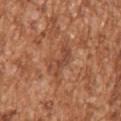Recorded during total-body skin imaging; not selected for excision or biopsy. A roughly 15 mm field-of-view crop from a total-body skin photograph. From the arm. A male patient about 45 years old. The recorded lesion diameter is about 4 mm.